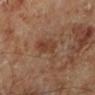Assessment: Captured during whole-body skin photography for melanoma surveillance; the lesion was not biopsied. Background: From the left lower leg. A close-up tile cropped from a whole-body skin photograph, about 15 mm across. The recorded lesion diameter is about 5 mm. A male subject, roughly 70 years of age.